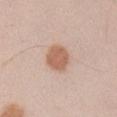This lesion was catalogued during total-body skin photography and was not selected for biopsy. A male subject, about 30 years old. Measured at roughly 3.5 mm in maximum diameter. A lesion tile, about 15 mm wide, cut from a 3D total-body photograph. From the right upper arm. Automated image analysis of the tile measured an average lesion color of about L≈61 a*≈21 b*≈30 (CIELAB), about 11 CIELAB-L* units darker than the surrounding skin, and a normalized border contrast of about 8.5. The analysis additionally found a border-irregularity rating of about 1.5/10, internal color variation of about 2 on a 0–10 scale, and peripheral color asymmetry of about 1.